A 15 mm close-up extracted from a 3D total-body photography capture. A male patient, aged 63–67. On the left upper arm: 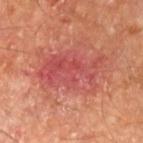Findings:
- histopathologic diagnosis — a nodular basal cell carcinoma